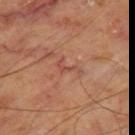Case summary:
– notes — no biopsy performed (imaged during a skin exam)
– lighting — cross-polarized illumination
– image — 15 mm crop, total-body photography
– automated lesion analysis — a shape eccentricity near 0.9 and two-axis asymmetry of about 0.55; a lesion color around L≈48 a*≈26 b*≈29 in CIELAB, about 7 CIELAB-L* units darker than the surrounding skin, and a normalized border contrast of about 5.5; a nevus-likeness score of about 0/100
– subject — aged approximately 65
– body site — the leg